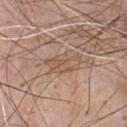workup = no biopsy performed (imaged during a skin exam) | illumination = white-light illumination | automated lesion analysis = a lesion area of about 7.5 mm², a shape eccentricity near 0.85, and two-axis asymmetry of about 0.35; a within-lesion color-variation index near 3/10 and radial color variation of about 1; a classifier nevus-likeness of about 0/100 | acquisition = ~15 mm tile from a whole-body skin photo | size = ~4.5 mm (longest diameter) | site = the chest | patient = male, aged 53 to 57.